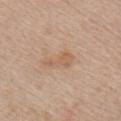  biopsy_status: not biopsied; imaged during a skin examination
  lighting: white-light
  site: right upper arm
  patient:
    sex: male
    age_approx: 60
  lesion_size:
    long_diameter_mm_approx: 4.0
  image:
    source: total-body photography crop
    field_of_view_mm: 15
  automated_metrics:
    area_mm2_approx: 5.0
    eccentricity: 0.9
    shape_asymmetry: 0.4